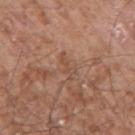notes — total-body-photography surveillance lesion; no biopsy
subject — male, approximately 55 years of age
image — ~15 mm crop, total-body skin-cancer survey
body site — the right upper arm
tile lighting — white-light illumination